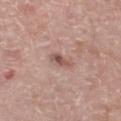biopsy_status: not biopsied; imaged during a skin examination
image:
  source: total-body photography crop
  field_of_view_mm: 15
patient:
  sex: male
  age_approx: 55
lighting: white-light
site: left lower leg
automated_metrics:
  border_irregularity_0_10: 3.5
  color_variation_0_10: 3.5
  peripheral_color_asymmetry: 1.0
  nevus_likeness_0_100: 5
  lesion_detection_confidence_0_100: 100
lesion_size:
  long_diameter_mm_approx: 2.5